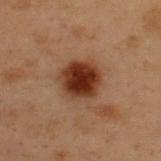The lesion was photographed on a routine skin check and not biopsied; there is no pathology result.
The lesion's longest dimension is about 4.5 mm.
The subject is a male in their mid- to late 50s.
The tile uses cross-polarized illumination.
Located on the upper back.
A 15 mm close-up tile from a total-body photography series done for melanoma screening.